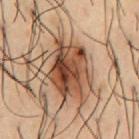Case summary:
* notes · imaged on a skin check; not biopsied
* imaging modality · total-body-photography crop, ~15 mm field of view
* body site · the chest
* patient · male, aged approximately 55
* tile lighting · cross-polarized illumination